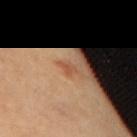This lesion was catalogued during total-body skin photography and was not selected for biopsy. A close-up tile cropped from a whole-body skin photograph, about 15 mm across. This is a cross-polarized tile. A female patient approximately 40 years of age. From the upper back. About 2 mm across.